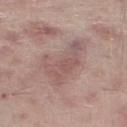| field | value |
|---|---|
| tile lighting | white-light |
| image | ~15 mm crop, total-body skin-cancer survey |
| location | the right lower leg |
| patient | male, in their mid- to late 60s |